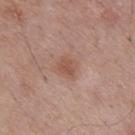The lesion was tiled from a total-body skin photograph and was not biopsied. A male patient in their 70s. About 2.5 mm across. A close-up tile cropped from a whole-body skin photograph, about 15 mm across. The tile uses white-light illumination. Automated image analysis of the tile measured a lesion area of about 4 mm², a shape eccentricity near 0.65, and two-axis asymmetry of about 0.25. It also reported an average lesion color of about L≈52 a*≈21 b*≈28 (CIELAB), roughly 8 lightness units darker than nearby skin, and a normalized lesion–skin contrast near 6. It also reported a classifier nevus-likeness of about 25/100. Located on the mid back.